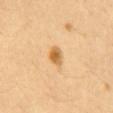<case>
<image>
  <source>total-body photography crop</source>
  <field_of_view_mm>15</field_of_view_mm>
</image>
<site>back</site>
<patient>
  <sex>female</sex>
  <age_approx>40</age_approx>
</patient>
</case>